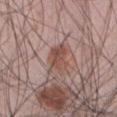Impression:
The lesion was photographed on a routine skin check and not biopsied; there is no pathology result.
Acquisition and patient details:
The lesion is on the front of the torso. Approximately 4 mm at its widest. A 15 mm close-up extracted from a 3D total-body photography capture. This is a white-light tile. A male subject aged around 45.